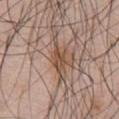Clinical impression:
The lesion was photographed on a routine skin check and not biopsied; there is no pathology result.
Clinical summary:
This is a white-light tile. Cropped from a total-body skin-imaging series; the visible field is about 15 mm. An algorithmic analysis of the crop reported a shape eccentricity near 0.8. The analysis additionally found a lesion color around L≈49 a*≈18 b*≈29 in CIELAB and a normalized border contrast of about 8. The software also gave border irregularity of about 3.5 on a 0–10 scale, internal color variation of about 2.5 on a 0–10 scale, and peripheral color asymmetry of about 1. It also reported a nevus-likeness score of about 20/100 and a lesion-detection confidence of about 60/100. Approximately 3 mm at its widest. On the front of the torso. A male subject, aged 68–72.